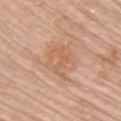biopsy status = no biopsy performed (imaged during a skin exam); site = the right upper arm; image-analysis metrics = a lesion color around L≈60 a*≈22 b*≈33 in CIELAB, a lesion–skin lightness drop of about 6, and a normalized border contrast of about 4.5; size = ≈4 mm; patient = female, approximately 65 years of age; image = 15 mm crop, total-body photography.